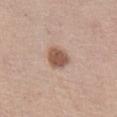Context: The lesion is located on the left thigh. A 15 mm close-up extracted from a 3D total-body photography capture. The subject is a female about 50 years old.